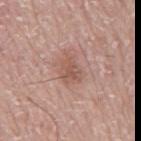Clinical impression: Imaged during a routine full-body skin examination; the lesion was not biopsied and no histopathology is available. Acquisition and patient details: A 15 mm crop from a total-body photograph taken for skin-cancer surveillance. The lesion is located on the mid back. A male patient, about 75 years old. This is a white-light tile. The recorded lesion diameter is about 3 mm.